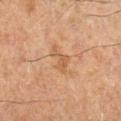follow-up: imaged on a skin check; not biopsied
patient: male, approximately 65 years of age
illumination: cross-polarized
imaging modality: ~15 mm tile from a whole-body skin photo
lesion size: ≈3.5 mm
body site: the right lower leg
TBP lesion metrics: a footprint of about 4.5 mm², an outline eccentricity of about 0.85 (0 = round, 1 = elongated), and two-axis asymmetry of about 0.4; a lesion–skin lightness drop of about 6; a lesion-detection confidence of about 100/100